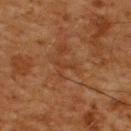– follow-up: catalogued during a skin exam; not biopsied
– subject: male, approximately 60 years of age
– diameter: ≈2.5 mm
– imaging modality: total-body-photography crop, ~15 mm field of view
– image-analysis metrics: a classifier nevus-likeness of about 0/100 and a detector confidence of about 100 out of 100 that the crop contains a lesion
– tile lighting: cross-polarized illumination
– site: the back